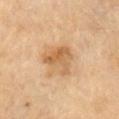The lesion was tiled from a total-body skin photograph and was not biopsied.
A male patient, in their 70s.
Automated tile analysis of the lesion measured an area of roughly 11 mm² and a shape eccentricity near 0.35.
Approximately 4 mm at its widest.
On the right upper arm.
Captured under cross-polarized illumination.
A 15 mm close-up tile from a total-body photography series done for melanoma screening.